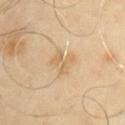The lesion is on the front of the torso. An algorithmic analysis of the crop reported a lesion area of about 5.5 mm² and a shape eccentricity near 0.3. The analysis additionally found a lesion color around L≈61 a*≈14 b*≈37 in CIELAB and a lesion-to-skin contrast of about 5.5 (normalized; higher = more distinct). The software also gave a nevus-likeness score of about 0/100 and lesion-presence confidence of about 100/100. Imaged with cross-polarized lighting. A male patient, aged approximately 65. The lesion's longest dimension is about 3 mm. A region of skin cropped from a whole-body photographic capture, roughly 15 mm wide.